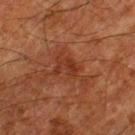No biopsy was performed on this lesion — it was imaged during a full skin examination and was not determined to be concerning. A male subject approximately 80 years of age. Cropped from a whole-body photographic skin survey; the tile spans about 15 mm. The lesion is on the left thigh. Automated tile analysis of the lesion measured an area of roughly 4 mm², a shape eccentricity near 0.7, and a shape-asymmetry score of about 0.55 (0 = symmetric). And it measured lesion-presence confidence of about 100/100.